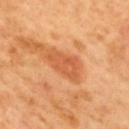Q: Was a biopsy performed?
A: total-body-photography surveillance lesion; no biopsy
Q: What is the anatomic site?
A: the mid back
Q: What kind of image is this?
A: 15 mm crop, total-body photography
Q: Automated lesion metrics?
A: a mean CIELAB color near L≈58 a*≈30 b*≈42 and about 9 CIELAB-L* units darker than the surrounding skin; lesion-presence confidence of about 100/100
Q: How was the tile lit?
A: cross-polarized
Q: Patient demographics?
A: male, roughly 50 years of age
Q: Lesion size?
A: ~5 mm (longest diameter)